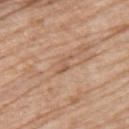Findings:
– body site: the upper back
– lesion diameter: about 3 mm
– patient: female, approximately 75 years of age
– illumination: white-light illumination
– acquisition: total-body-photography crop, ~15 mm field of view